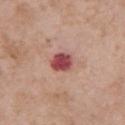Part of a total-body skin-imaging series; this lesion was reviewed on a skin check and was not flagged for biopsy. Imaged with white-light lighting. The lesion-visualizer software estimated an average lesion color of about L≈48 a*≈32 b*≈23 (CIELAB), a lesion–skin lightness drop of about 17, and a normalized border contrast of about 11.5. The subject is a female about 55 years old. The recorded lesion diameter is about 2.5 mm. A 15 mm crop from a total-body photograph taken for skin-cancer surveillance. The lesion is located on the right upper arm.